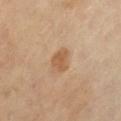biopsy status — imaged on a skin check; not biopsied | subject — male, approximately 65 years of age | body site — the abdomen | acquisition — ~15 mm tile from a whole-body skin photo | lighting — cross-polarized illumination.